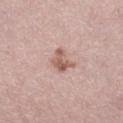A roughly 15 mm field-of-view crop from a total-body skin photograph. Approximately 3 mm at its widest. The lesion is on the lower back. The tile uses white-light illumination. The total-body-photography lesion software estimated a shape eccentricity near 0.7. The software also gave a lesion–skin lightness drop of about 11 and a lesion-to-skin contrast of about 7.5 (normalized; higher = more distinct). The software also gave internal color variation of about 3.5 on a 0–10 scale and a peripheral color-asymmetry measure near 1.5. It also reported a nevus-likeness score of about 20/100. A female subject aged 48 to 52.